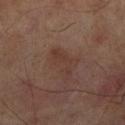Q: Was a biopsy performed?
A: no biopsy performed (imaged during a skin exam)
Q: How was this image acquired?
A: total-body-photography crop, ~15 mm field of view
Q: What are the patient's age and sex?
A: male, aged 68 to 72
Q: What lighting was used for the tile?
A: cross-polarized illumination
Q: Lesion location?
A: the right lower leg